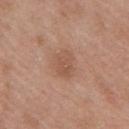The lesion was photographed on a routine skin check and not biopsied; there is no pathology result.